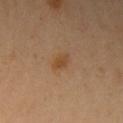Clinical impression: Captured during whole-body skin photography for melanoma surveillance; the lesion was not biopsied. Background: A 15 mm crop from a total-body photograph taken for skin-cancer surveillance. The lesion is on the arm. The subject is a female aged 48 to 52. This is a cross-polarized tile. Approximately 3 mm at its widest.